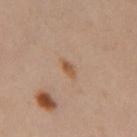<case>
  <lighting>cross-polarized</lighting>
  <site>left thigh</site>
  <lesion_size>
    <long_diameter_mm_approx>2.5</long_diameter_mm_approx>
  </lesion_size>
  <image>
    <source>total-body photography crop</source>
    <field_of_view_mm>15</field_of_view_mm>
  </image>
  <patient>
    <sex>female</sex>
    <age_approx>40</age_approx>
  </patient>
</case>